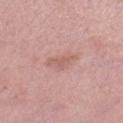Assessment:
Part of a total-body skin-imaging series; this lesion was reviewed on a skin check and was not flagged for biopsy.
Context:
A female patient aged approximately 40. This image is a 15 mm lesion crop taken from a total-body photograph. On the left lower leg.